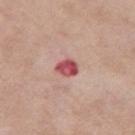Q: Is there a histopathology result?
A: no biopsy performed (imaged during a skin exam)
Q: What did automated image analysis measure?
A: a lesion area of about 4.5 mm²; a border-irregularity index near 1/10 and radial color variation of about 1.5
Q: What is the anatomic site?
A: the leg
Q: What lighting was used for the tile?
A: white-light
Q: How was this image acquired?
A: total-body-photography crop, ~15 mm field of view
Q: Patient demographics?
A: female, roughly 55 years of age